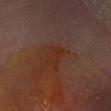No biopsy was performed on this lesion — it was imaged during a full skin examination and was not determined to be concerning.
Automated image analysis of the tile measured a lesion–skin lightness drop of about 3 and a normalized lesion–skin contrast near 7.
This image is a 15 mm lesion crop taken from a total-body photograph.
The tile uses cross-polarized illumination.
The patient is a male aged approximately 50.
Located on the left leg.